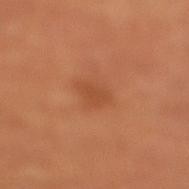Impression:
Recorded during total-body skin imaging; not selected for excision or biopsy.
Acquisition and patient details:
The recorded lesion diameter is about 2.5 mm. A male patient, in their 50s. On the right lower leg. This is a cross-polarized tile. This image is a 15 mm lesion crop taken from a total-body photograph.